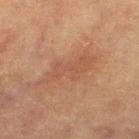notes — catalogued during a skin exam; not biopsied | body site — the leg | image source — total-body-photography crop, ~15 mm field of view | subject — female, aged 78 to 82.